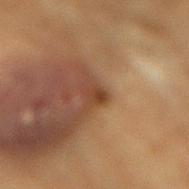Recorded during total-body skin imaging; not selected for excision or biopsy.
Captured under cross-polarized illumination.
A male patient aged approximately 85 or older.
The recorded lesion diameter is about 2.5 mm.
From the mid back.
A lesion tile, about 15 mm wide, cut from a 3D total-body photograph.